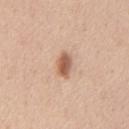location: the chest
subject: male, aged approximately 45
tile lighting: white-light
image source: ~15 mm tile from a whole-body skin photo
lesion size: about 3 mm
TBP lesion metrics: a lesion color around L≈59 a*≈21 b*≈32 in CIELAB, a lesion–skin lightness drop of about 14, and a normalized border contrast of about 9; a border-irregularity rating of about 2/10, a color-variation rating of about 3.5/10, and peripheral color asymmetry of about 1; lesion-presence confidence of about 100/100